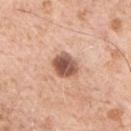Imaged during a routine full-body skin examination; the lesion was not biopsied and no histopathology is available. Cropped from a total-body skin-imaging series; the visible field is about 15 mm. The lesion is located on the chest. Automated image analysis of the tile measured a lesion area of about 7 mm² and a symmetry-axis asymmetry near 0.2. It also reported a mean CIELAB color near L≈55 a*≈22 b*≈29, roughly 17 lightness units darker than nearby skin, and a normalized border contrast of about 11. And it measured border irregularity of about 1.5 on a 0–10 scale and a color-variation rating of about 5.5/10. And it measured a classifier nevus-likeness of about 40/100 and a detector confidence of about 100 out of 100 that the crop contains a lesion. Captured under white-light illumination. Longest diameter approximately 3.5 mm. A male subject, in their 70s.